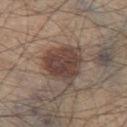{"biopsy_status": "not biopsied; imaged during a skin examination", "site": "left thigh", "patient": {"sex": "male", "age_approx": 45}, "image": {"source": "total-body photography crop", "field_of_view_mm": 15}, "lighting": "white-light"}